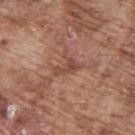biopsy_status: not biopsied; imaged during a skin examination
automated_metrics:
  area_mm2_approx: 3.5
  eccentricity: 0.85
  shape_asymmetry: 0.5
  cielab_L: 47
  cielab_a: 22
  cielab_b: 27
  vs_skin_contrast_norm: 6.0
  border_irregularity_0_10: 5.5
  color_variation_0_10: 1.5
  peripheral_color_asymmetry: 0.5
lighting: white-light
image:
  source: total-body photography crop
  field_of_view_mm: 15
site: upper back
patient:
  sex: male
  age_approx: 75
lesion_size:
  long_diameter_mm_approx: 3.0